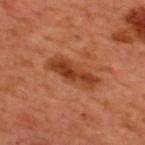| feature | finding |
|---|---|
| follow-up | no biopsy performed (imaged during a skin exam) |
| automated lesion analysis | a lesion color around L≈41 a*≈30 b*≈37 in CIELAB, roughly 11 lightness units darker than nearby skin, and a lesion-to-skin contrast of about 9 (normalized; higher = more distinct); border irregularity of about 3.5 on a 0–10 scale; a classifier nevus-likeness of about 30/100 |
| image source | 15 mm crop, total-body photography |
| lesion size | about 6 mm |
| location | the upper back |
| subject | male, in their 50s |